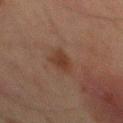follow-up: no biopsy performed (imaged during a skin exam)
site: the abdomen
image source: ~15 mm tile from a whole-body skin photo
patient: male, aged approximately 65